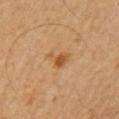Context:
An algorithmic analysis of the crop reported an eccentricity of roughly 0.65. The analysis additionally found an average lesion color of about L≈52 a*≈21 b*≈39 (CIELAB), about 9 CIELAB-L* units darker than the surrounding skin, and a normalized border contrast of about 7.5. A region of skin cropped from a whole-body photographic capture, roughly 15 mm wide. The lesion's longest dimension is about 3 mm. The lesion is on the left upper arm. The tile uses cross-polarized illumination. The subject is a male aged approximately 60.